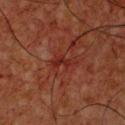Q: What are the patient's age and sex?
A: male, approximately 65 years of age
Q: What is the lesion's diameter?
A: ~3 mm (longest diameter)
Q: What lighting was used for the tile?
A: cross-polarized illumination
Q: Where on the body is the lesion?
A: the chest
Q: How was this image acquired?
A: ~15 mm crop, total-body skin-cancer survey